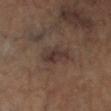Imaged during a routine full-body skin examination; the lesion was not biopsied and no histopathology is available.
Captured under cross-polarized illumination.
A 15 mm crop from a total-body photograph taken for skin-cancer surveillance.
On the left lower leg.
The lesion's longest dimension is about 4 mm.
The subject is a female in their mid- to late 60s.